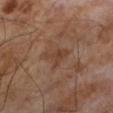Findings:
- notes: no biopsy performed (imaged during a skin exam)
- image source: 15 mm crop, total-body photography
- automated lesion analysis: a classifier nevus-likeness of about 0/100 and lesion-presence confidence of about 100/100
- subject: male, aged around 65
- lesion diameter: ≈3 mm
- lighting: cross-polarized